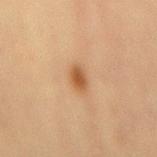About 2.5 mm across.
A roughly 15 mm field-of-view crop from a total-body skin photograph.
A female subject in their mid-60s.
Automated tile analysis of the lesion measured an eccentricity of roughly 0.8 and a symmetry-axis asymmetry near 0.2. The analysis additionally found a lesion color around L≈47 a*≈20 b*≈34 in CIELAB and a normalized border contrast of about 8.5. And it measured a border-irregularity index near 1.5/10, internal color variation of about 2 on a 0–10 scale, and a peripheral color-asymmetry measure near 0.5. The software also gave a classifier nevus-likeness of about 95/100 and a lesion-detection confidence of about 100/100.
Imaged with cross-polarized lighting.
On the mid back.